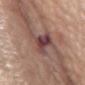<tbp_lesion>
  <image>
    <source>total-body photography crop</source>
    <field_of_view_mm>15</field_of_view_mm>
  </image>
  <site>chest</site>
  <patient>
    <sex>male</sex>
    <age_approx>75</age_approx>
  </patient>
</tbp_lesion>